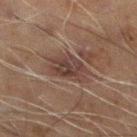Impression:
Part of a total-body skin-imaging series; this lesion was reviewed on a skin check and was not flagged for biopsy.
Clinical summary:
The subject is a male aged 58–62. The recorded lesion diameter is about 4.5 mm. The total-body-photography lesion software estimated a footprint of about 12 mm² and a shape eccentricity near 0.7. And it measured a border-irregularity index near 3.5/10 and a color-variation rating of about 4/10. It also reported a classifier nevus-likeness of about 0/100 and a detector confidence of about 75 out of 100 that the crop contains a lesion. On the left lower leg. Cropped from a total-body skin-imaging series; the visible field is about 15 mm.